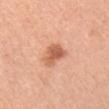notes = catalogued during a skin exam; not biopsied | illumination = white-light illumination | patient = female, roughly 45 years of age | anatomic site = the left upper arm | size = ≈3 mm | acquisition = ~15 mm tile from a whole-body skin photo.